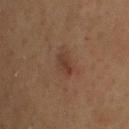Findings:
- biopsy status: total-body-photography surveillance lesion; no biopsy
- lighting: cross-polarized
- image source: total-body-photography crop, ~15 mm field of view
- patient: male, in their 60s
- lesion diameter: about 2.5 mm
- image-analysis metrics: an eccentricity of roughly 0.8 and a shape-asymmetry score of about 0.2 (0 = symmetric); a mean CIELAB color near L≈32 a*≈17 b*≈24, a lesion–skin lightness drop of about 6, and a normalized border contrast of about 6; a classifier nevus-likeness of about 20/100